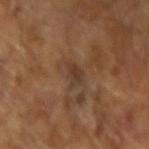Q: Was a biopsy performed?
A: imaged on a skin check; not biopsied
Q: What is the imaging modality?
A: 15 mm crop, total-body photography
Q: What are the patient's age and sex?
A: male, aged 63 to 67
Q: How large is the lesion?
A: ~2.5 mm (longest diameter)
Q: How was the tile lit?
A: cross-polarized illumination